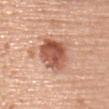No biopsy was performed on this lesion — it was imaged during a full skin examination and was not determined to be concerning.
Imaged with white-light lighting.
The lesion is on the upper back.
The lesion's longest dimension is about 5 mm.
A female subject in their 60s.
A roughly 15 mm field-of-view crop from a total-body skin photograph.